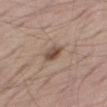Assessment: Imaged during a routine full-body skin examination; the lesion was not biopsied and no histopathology is available. Clinical summary: Imaged with white-light lighting. A male subject roughly 55 years of age. Cropped from a total-body skin-imaging series; the visible field is about 15 mm. The lesion is located on the right thigh. The recorded lesion diameter is about 2.5 mm.